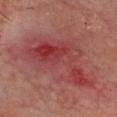<record>
  <lesion_size>
    <long_diameter_mm_approx>9.0</long_diameter_mm_approx>
  </lesion_size>
  <patient>
    <sex>male</sex>
    <age_approx>65</age_approx>
  </patient>
  <image>
    <source>total-body photography crop</source>
    <field_of_view_mm>15</field_of_view_mm>
  </image>
  <lighting>cross-polarized</lighting>
  <site>head or neck</site>
</record>